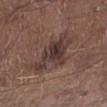Q: Was a biopsy performed?
A: imaged on a skin check; not biopsied
Q: What are the patient's age and sex?
A: male, in their mid-50s
Q: Illumination type?
A: white-light illumination
Q: What is the lesion's diameter?
A: ≈6 mm
Q: Lesion location?
A: the left lower leg
Q: How was this image acquired?
A: total-body-photography crop, ~15 mm field of view
Q: Automated lesion metrics?
A: a lesion area of about 16 mm², an eccentricity of roughly 0.8, and two-axis asymmetry of about 0.3; an average lesion color of about L≈36 a*≈16 b*≈18 (CIELAB), roughly 9 lightness units darker than nearby skin, and a normalized border contrast of about 9; lesion-presence confidence of about 100/100